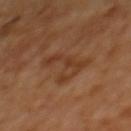Q: How was this image acquired?
A: ~15 mm crop, total-body skin-cancer survey
Q: Lesion location?
A: the upper back
Q: What are the patient's age and sex?
A: female, roughly 40 years of age
Q: Lesion size?
A: ~6 mm (longest diameter)